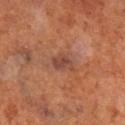Q: Was a biopsy performed?
A: catalogued during a skin exam; not biopsied
Q: How large is the lesion?
A: ≈3 mm
Q: How was this image acquired?
A: 15 mm crop, total-body photography
Q: Lesion location?
A: the right lower leg
Q: Patient demographics?
A: male, approximately 70 years of age
Q: Illumination type?
A: cross-polarized